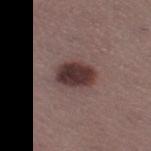Q: Was this lesion biopsied?
A: imaged on a skin check; not biopsied
Q: What kind of image is this?
A: total-body-photography crop, ~15 mm field of view
Q: What are the patient's age and sex?
A: female, aged approximately 55
Q: Where on the body is the lesion?
A: the right thigh
Q: What did automated image analysis measure?
A: a border-irregularity index near 2/10; an automated nevus-likeness rating near 95 out of 100 and a lesion-detection confidence of about 100/100
Q: How was the tile lit?
A: white-light illumination
Q: Lesion size?
A: ~4 mm (longest diameter)